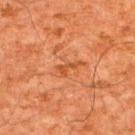biopsy status: imaged on a skin check; not biopsied | imaging modality: ~15 mm tile from a whole-body skin photo | diameter: about 3 mm | site: the upper back | subject: male, aged 58 to 62 | illumination: cross-polarized.